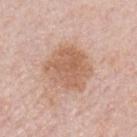Q: Is there a histopathology result?
A: total-body-photography surveillance lesion; no biopsy
Q: Automated lesion metrics?
A: an area of roughly 19 mm², an eccentricity of roughly 0.45, and two-axis asymmetry of about 0.15; border irregularity of about 2 on a 0–10 scale and radial color variation of about 1; a classifier nevus-likeness of about 0/100 and a lesion-detection confidence of about 100/100
Q: How was this image acquired?
A: total-body-photography crop, ~15 mm field of view
Q: Lesion size?
A: ≈5 mm
Q: Where on the body is the lesion?
A: the chest
Q: Who is the patient?
A: male, about 60 years old
Q: What lighting was used for the tile?
A: white-light illumination